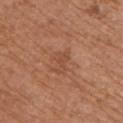Clinical impression: Recorded during total-body skin imaging; not selected for excision or biopsy. Context: The lesion is on the upper back. Approximately 2.5 mm at its widest. A 15 mm close-up extracted from a 3D total-body photography capture. The tile uses white-light illumination. A male subject, approximately 70 years of age.